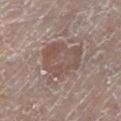Assessment: Captured during whole-body skin photography for melanoma surveillance; the lesion was not biopsied. Background: A roughly 15 mm field-of-view crop from a total-body skin photograph. A male subject, aged 78 to 82. On the right leg.